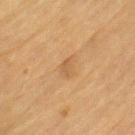follow-up: total-body-photography surveillance lesion; no biopsy | automated metrics: a lesion area of about 3.5 mm² and a shape eccentricity near 0.75 | lesion size: ~2.5 mm (longest diameter) | tile lighting: cross-polarized illumination | subject: male, about 85 years old or older | image: ~15 mm crop, total-body skin-cancer survey | site: the left upper arm.